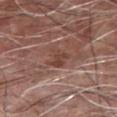- workup — imaged on a skin check; not biopsied
- illumination — white-light
- acquisition — total-body-photography crop, ~15 mm field of view
- image-analysis metrics — a lesion color around L≈43 a*≈21 b*≈25 in CIELAB, a lesion–skin lightness drop of about 7, and a lesion-to-skin contrast of about 6 (normalized; higher = more distinct)
- subject — male, in their mid- to late 60s
- body site — the chest
- lesion size — ≈2.5 mm